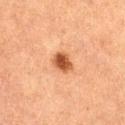This lesion was catalogued during total-body skin photography and was not selected for biopsy.
A lesion tile, about 15 mm wide, cut from a 3D total-body photograph.
The lesion is on the left thigh.
A female patient, aged 58–62.